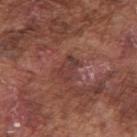Captured during whole-body skin photography for melanoma surveillance; the lesion was not biopsied. The lesion is on the right upper arm. A male patient, aged approximately 75. Imaged with white-light lighting. Measured at roughly 3 mm in maximum diameter. Cropped from a total-body skin-imaging series; the visible field is about 15 mm.